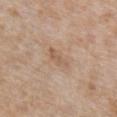– workup · imaged on a skin check; not biopsied
– image source · 15 mm crop, total-body photography
– patient · male, aged approximately 65
– location · the right upper arm